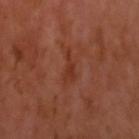No biopsy was performed on this lesion — it was imaged during a full skin examination and was not determined to be concerning. The lesion's longest dimension is about 4 mm. A 15 mm close-up tile from a total-body photography series done for melanoma screening. The tile uses cross-polarized illumination. A male patient, roughly 55 years of age. On the head or neck. An algorithmic analysis of the crop reported an area of roughly 3.5 mm², an outline eccentricity of about 0.95 (0 = round, 1 = elongated), and a symmetry-axis asymmetry near 0.55. And it measured an average lesion color of about L≈35 a*≈27 b*≈31 (CIELAB) and a lesion–skin lightness drop of about 6. And it measured a classifier nevus-likeness of about 0/100 and a detector confidence of about 95 out of 100 that the crop contains a lesion.